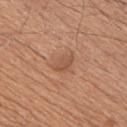{
  "biopsy_status": "not biopsied; imaged during a skin examination",
  "lighting": "white-light",
  "image": {
    "source": "total-body photography crop",
    "field_of_view_mm": 15
  },
  "lesion_size": {
    "long_diameter_mm_approx": 3.0
  },
  "site": "chest",
  "patient": {
    "sex": "male",
    "age_approx": 60
  },
  "automated_metrics": {
    "cielab_L": 53,
    "cielab_a": 22,
    "cielab_b": 31,
    "vs_skin_darker_L": 7.0,
    "vs_skin_contrast_norm": 5.5,
    "border_irregularity_0_10": 2.0,
    "color_variation_0_10": 2.0,
    "peripheral_color_asymmetry": 0.5
  }
}